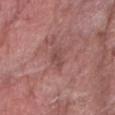Notes:
• biopsy status: imaged on a skin check; not biopsied
• image: ~15 mm crop, total-body skin-cancer survey
• subject: male, aged 78 to 82
• illumination: white-light illumination
• anatomic site: the right forearm